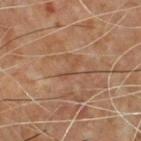notes=no biopsy performed (imaged during a skin exam).